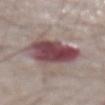{
  "biopsy_status": "not biopsied; imaged during a skin examination",
  "lesion_size": {
    "long_diameter_mm_approx": 6.5
  },
  "patient": {
    "sex": "male",
    "age_approx": 75
  },
  "image": {
    "source": "total-body photography crop",
    "field_of_view_mm": 15
  },
  "site": "abdomen"
}